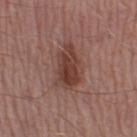Q: Was a biopsy performed?
A: no biopsy performed (imaged during a skin exam)